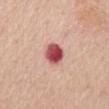The lesion was tiled from a total-body skin photograph and was not biopsied. On the chest. A lesion tile, about 15 mm wide, cut from a 3D total-body photograph. A male patient, aged 58–62.